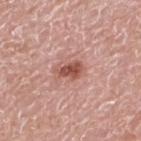This lesion was catalogued during total-body skin photography and was not selected for biopsy.
From the mid back.
Automated tile analysis of the lesion measured a lesion color around L≈52 a*≈26 b*≈27 in CIELAB, a lesion–skin lightness drop of about 13, and a lesion-to-skin contrast of about 9 (normalized; higher = more distinct). The software also gave border irregularity of about 3 on a 0–10 scale and peripheral color asymmetry of about 1. And it measured lesion-presence confidence of about 100/100.
A male patient, aged around 75.
A 15 mm close-up extracted from a 3D total-body photography capture.